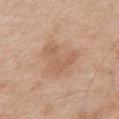notes = total-body-photography surveillance lesion; no biopsy | body site = the chest | image = ~15 mm tile from a whole-body skin photo | lesion diameter = ≈4.5 mm | patient = male, in their mid- to late 60s | lighting = white-light | automated lesion analysis = a lesion area of about 13 mm², a shape eccentricity near 0.5, and a symmetry-axis asymmetry near 0.45; a mean CIELAB color near L≈60 a*≈19 b*≈32, about 7 CIELAB-L* units darker than the surrounding skin, and a normalized lesion–skin contrast near 5.